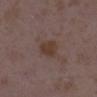Case summary:
* biopsy status: total-body-photography surveillance lesion; no biopsy
* patient: female, approximately 35 years of age
* acquisition: ~15 mm crop, total-body skin-cancer survey
* tile lighting: white-light
* body site: the right lower leg
* size: ~3 mm (longest diameter)